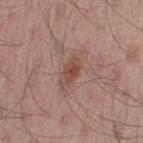Captured during whole-body skin photography for melanoma surveillance; the lesion was not biopsied.
The patient is a male aged 53 to 57.
Captured under white-light illumination.
A close-up tile cropped from a whole-body skin photograph, about 15 mm across.
Automated tile analysis of the lesion measured a lesion color around L≈47 a*≈20 b*≈25 in CIELAB. And it measured a nevus-likeness score of about 40/100 and a detector confidence of about 100 out of 100 that the crop contains a lesion.
Approximately 3.5 mm at its widest.
From the left thigh.